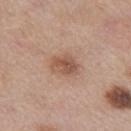No biopsy was performed on this lesion — it was imaged during a full skin examination and was not determined to be concerning.
A 15 mm close-up tile from a total-body photography series done for melanoma screening.
A female subject, approximately 40 years of age.
On the leg.